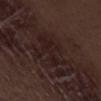{
  "site": "left thigh",
  "image": {
    "source": "total-body photography crop",
    "field_of_view_mm": 15
  },
  "patient": {
    "sex": "male",
    "age_approx": 70
  }
}